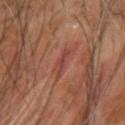| field | value |
|---|---|
| image source | 15 mm crop, total-body photography |
| patient | male, aged approximately 65 |
| image-analysis metrics | a lesion area of about 3 mm², a shape eccentricity near 0.95, and a symmetry-axis asymmetry near 0.4; a border-irregularity rating of about 5/10, a within-lesion color-variation index near 0/10, and a peripheral color-asymmetry measure near 0; a nevus-likeness score of about 0/100 and lesion-presence confidence of about 70/100 |
| anatomic site | the arm |
| illumination | cross-polarized |